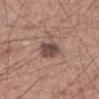Q: Was a biopsy performed?
A: imaged on a skin check; not biopsied
Q: Lesion location?
A: the upper back
Q: What kind of image is this?
A: total-body-photography crop, ~15 mm field of view
Q: Patient demographics?
A: male, aged around 60
Q: Lesion size?
A: ≈3.5 mm